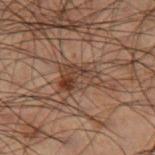biopsy status = imaged on a skin check; not biopsied
site = the right thigh
lighting = cross-polarized illumination
image = ~15 mm tile from a whole-body skin photo
patient = male, approximately 50 years of age
lesion size = ≈4.5 mm
image-analysis metrics = an area of roughly 11 mm² and a shape eccentricity near 0.75; an automated nevus-likeness rating near 20 out of 100 and lesion-presence confidence of about 95/100